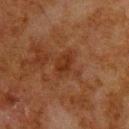| field | value |
|---|---|
| follow-up | total-body-photography surveillance lesion; no biopsy |
| site | the upper back |
| subject | male, in their 80s |
| image source | total-body-photography crop, ~15 mm field of view |